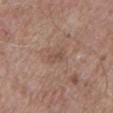Part of a total-body skin-imaging series; this lesion was reviewed on a skin check and was not flagged for biopsy.
A male patient, in their mid- to late 80s or older.
Cropped from a whole-body photographic skin survey; the tile spans about 15 mm.
The lesion is located on the left lower leg.
Captured under white-light illumination.
An algorithmic analysis of the crop reported a lesion area of about 2.5 mm², a shape eccentricity near 0.85, and a shape-asymmetry score of about 0.35 (0 = symmetric). The software also gave a color-variation rating of about 0/10 and a peripheral color-asymmetry measure near 0.